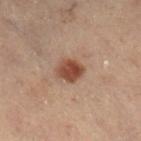On the leg. A male subject, aged 53 to 57. A roughly 15 mm field-of-view crop from a total-body skin photograph. The tile uses cross-polarized illumination.